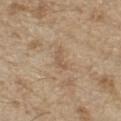Q: Was this lesion biopsied?
A: no biopsy performed (imaged during a skin exam)
Q: How was this image acquired?
A: ~15 mm tile from a whole-body skin photo
Q: Automated lesion metrics?
A: a lesion area of about 2.5 mm² and two-axis asymmetry of about 0.5; an average lesion color of about L≈56 a*≈15 b*≈31 (CIELAB) and a normalized border contrast of about 5; internal color variation of about 0 on a 0–10 scale and a peripheral color-asymmetry measure near 0; lesion-presence confidence of about 100/100
Q: Who is the patient?
A: male, aged around 70
Q: What lighting was used for the tile?
A: white-light illumination
Q: Lesion location?
A: the chest